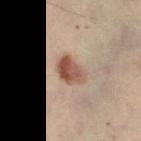Captured during whole-body skin photography for melanoma surveillance; the lesion was not biopsied. The subject is a female aged around 60. From the left thigh. Measured at roughly 3.5 mm in maximum diameter. Cropped from a total-body skin-imaging series; the visible field is about 15 mm. Captured under cross-polarized illumination.